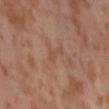No biopsy was performed on this lesion — it was imaged during a full skin examination and was not determined to be concerning.
Cropped from a total-body skin-imaging series; the visible field is about 15 mm.
The lesion is on the lower back.
The patient is a female aged around 55.
Captured under cross-polarized illumination.
The lesion's longest dimension is about 3 mm.